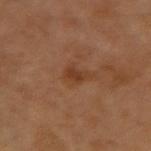No biopsy was performed on this lesion — it was imaged during a full skin examination and was not determined to be concerning.
The lesion is located on the left arm.
A roughly 15 mm field-of-view crop from a total-body skin photograph.
Captured under cross-polarized illumination.
The recorded lesion diameter is about 2.5 mm.
Automated tile analysis of the lesion measured an area of roughly 3.5 mm² and a shape-asymmetry score of about 0.3 (0 = symmetric).
The subject is a male aged 63–67.